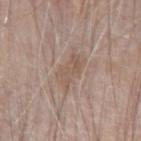<lesion>
  <biopsy_status>not biopsied; imaged during a skin examination</biopsy_status>
  <patient>
    <sex>male</sex>
    <age_approx>70</age_approx>
  </patient>
  <site>left forearm</site>
  <image>
    <source>total-body photography crop</source>
    <field_of_view_mm>15</field_of_view_mm>
  </image>
  <automated_metrics>
    <area_mm2_approx>8.0</area_mm2_approx>
    <eccentricity>0.85</eccentricity>
    <shape_asymmetry>0.3</shape_asymmetry>
    <border_irregularity_0_10>4.0</border_irregularity_0_10>
    <color_variation_0_10>2.5</color_variation_0_10>
    <peripheral_color_asymmetry>1.0</peripheral_color_asymmetry>
    <nevus_likeness_0_100>0</nevus_likeness_0_100>
    <lesion_detection_confidence_0_100>95</lesion_detection_confidence_0_100>
  </automated_metrics>
  <lesion_size>
    <long_diameter_mm_approx>4.0</long_diameter_mm_approx>
  </lesion_size>
  <lighting>white-light</lighting>
</lesion>